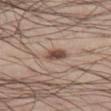<lesion>
<site>left thigh</site>
<lighting>white-light</lighting>
<image>
  <source>total-body photography crop</source>
  <field_of_view_mm>15</field_of_view_mm>
</image>
<patient>
  <sex>male</sex>
  <age_approx>55</age_approx>
</patient>
</lesion>